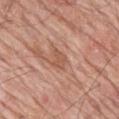Assessment:
Part of a total-body skin-imaging series; this lesion was reviewed on a skin check and was not flagged for biopsy.
Background:
The subject is a male aged 78 to 82. The lesion is located on the mid back. A lesion tile, about 15 mm wide, cut from a 3D total-body photograph. The total-body-photography lesion software estimated a mean CIELAB color near L≈55 a*≈23 b*≈31 and roughly 8 lightness units darker than nearby skin. The software also gave a classifier nevus-likeness of about 0/100 and lesion-presence confidence of about 100/100.